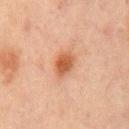Acquisition and patient details:
Automated image analysis of the tile measured a lesion color around L≈47 a*≈21 b*≈31 in CIELAB and a lesion–skin lightness drop of about 10. The software also gave a nevus-likeness score of about 95/100 and lesion-presence confidence of about 100/100. A male patient, roughly 65 years of age. A region of skin cropped from a whole-body photographic capture, roughly 15 mm wide. Longest diameter approximately 3.5 mm. Captured under cross-polarized illumination. Located on the mid back.